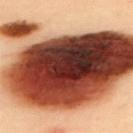Assessment:
Captured during whole-body skin photography for melanoma surveillance; the lesion was not biopsied.
Context:
Imaged with cross-polarized lighting. A female patient aged around 60. A roughly 15 mm field-of-view crop from a total-body skin photograph. The lesion is located on the upper back.